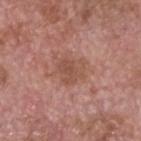biopsy status — total-body-photography surveillance lesion; no biopsy | tile lighting — white-light illumination | automated metrics — border irregularity of about 3.5 on a 0–10 scale, a color-variation rating of about 2.5/10, and peripheral color asymmetry of about 1 | lesion diameter — about 4 mm | body site — the head or neck | patient — male, aged 73–77 | acquisition — ~15 mm crop, total-body skin-cancer survey.